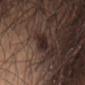No biopsy was performed on this lesion — it was imaged during a full skin examination and was not determined to be concerning.
A male patient in their mid-30s.
The lesion's longest dimension is about 2.5 mm.
A 15 mm close-up extracted from a 3D total-body photography capture.
Located on the front of the torso.
Captured under white-light illumination.
Automated tile analysis of the lesion measured radial color variation of about 0.5.